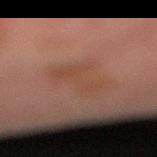Assessment: Captured during whole-body skin photography for melanoma surveillance; the lesion was not biopsied. Context: This image is a 15 mm lesion crop taken from a total-body photograph. From the leg. This is a cross-polarized tile. Measured at roughly 4.5 mm in maximum diameter. A male subject, aged 73–77.